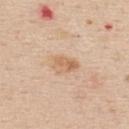An algorithmic analysis of the crop reported a footprint of about 5.5 mm², an outline eccentricity of about 0.75 (0 = round, 1 = elongated), and a shape-asymmetry score of about 0.35 (0 = symmetric).
About 3 mm across.
On the upper back.
The tile uses white-light illumination.
The subject is a female aged approximately 45.
A lesion tile, about 15 mm wide, cut from a 3D total-body photograph.